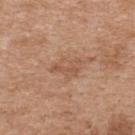Recorded during total-body skin imaging; not selected for excision or biopsy.
A female subject roughly 40 years of age.
From the upper back.
A 15 mm close-up tile from a total-body photography series done for melanoma screening.